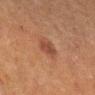Assessment:
Captured during whole-body skin photography for melanoma surveillance; the lesion was not biopsied.
Clinical summary:
Located on the right lower leg. Approximately 2.5 mm at its widest. A 15 mm close-up tile from a total-body photography series done for melanoma screening. Captured under cross-polarized illumination. A female subject aged around 55.